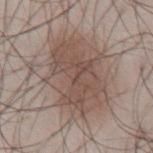| key | value |
|---|---|
| notes | no biopsy performed (imaged during a skin exam) |
| TBP lesion metrics | an average lesion color of about L≈50 a*≈16 b*≈23 (CIELAB), a lesion–skin lightness drop of about 9, and a normalized lesion–skin contrast near 7 |
| imaging modality | ~15 mm tile from a whole-body skin photo |
| size | ≈8 mm |
| illumination | white-light |
| patient | male, in their mid-40s |
| site | the front of the torso |